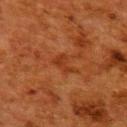Recorded during total-body skin imaging; not selected for excision or biopsy.
From the back.
Captured under cross-polarized illumination.
A female patient, aged approximately 50.
This image is a 15 mm lesion crop taken from a total-body photograph.